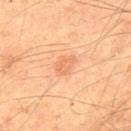workup: catalogued during a skin exam; not biopsied | patient: male, about 65 years old | lighting: cross-polarized | site: the upper back | lesion size: about 2.5 mm | automated metrics: a lesion area of about 4 mm², an outline eccentricity of about 0.75 (0 = round, 1 = elongated), and two-axis asymmetry of about 0.3; a lesion–skin lightness drop of about 8 and a lesion-to-skin contrast of about 4.5 (normalized; higher = more distinct); border irregularity of about 3 on a 0–10 scale and a color-variation rating of about 2/10 | image: ~15 mm crop, total-body skin-cancer survey.